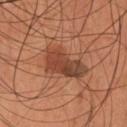The lesion was photographed on a routine skin check and not biopsied; there is no pathology result.
A male subject in their 40s.
Located on the head or neck.
About 6 mm across.
The lesion-visualizer software estimated a lesion area of about 13 mm², an outline eccentricity of about 0.85 (0 = round, 1 = elongated), and two-axis asymmetry of about 0.35. The analysis additionally found a border-irregularity rating of about 4/10.
A roughly 15 mm field-of-view crop from a total-body skin photograph.
Imaged with cross-polarized lighting.